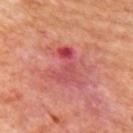notes = total-body-photography surveillance lesion; no biopsy.